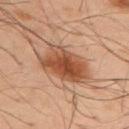The lesion was tiled from a total-body skin photograph and was not biopsied. On the back. Longest diameter approximately 6.5 mm. The patient is a male aged 48–52. A roughly 15 mm field-of-view crop from a total-body skin photograph.